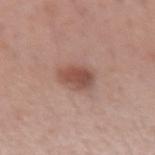Q: Was a biopsy performed?
A: total-body-photography surveillance lesion; no biopsy
Q: How large is the lesion?
A: about 4 mm
Q: What lighting was used for the tile?
A: white-light
Q: Who is the patient?
A: male, aged around 40
Q: Lesion location?
A: the right forearm
Q: How was this image acquired?
A: ~15 mm crop, total-body skin-cancer survey